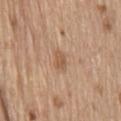workup = total-body-photography surveillance lesion; no biopsy
imaging modality = total-body-photography crop, ~15 mm field of view
subject = male, about 70 years old
anatomic site = the mid back